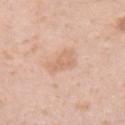| feature | finding |
|---|---|
| workup | no biopsy performed (imaged during a skin exam) |
| site | the right upper arm |
| image | ~15 mm tile from a whole-body skin photo |
| subject | female, about 30 years old |